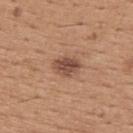biopsy status: total-body-photography surveillance lesion; no biopsy